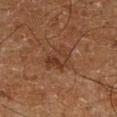<case>
<biopsy_status>not biopsied; imaged during a skin examination</biopsy_status>
<automated_metrics>
  <area_mm2_approx>8.5</area_mm2_approx>
  <eccentricity>0.65</eccentricity>
  <border_irregularity_0_10>3.0</border_irregularity_0_10>
  <color_variation_0_10>4.5</color_variation_0_10>
  <peripheral_color_asymmetry>2.0</peripheral_color_asymmetry>
  <nevus_likeness_0_100>0</nevus_likeness_0_100>
</automated_metrics>
<lighting>cross-polarized</lighting>
<lesion_size>
  <long_diameter_mm_approx>4.0</long_diameter_mm_approx>
</lesion_size>
<image>
  <source>total-body photography crop</source>
  <field_of_view_mm>15</field_of_view_mm>
</image>
<patient>
  <sex>male</sex>
  <age_approx>65</age_approx>
</patient>
<site>right lower leg</site>
</case>